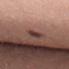Impression: Captured during whole-body skin photography for melanoma surveillance; the lesion was not biopsied. Context: Approximately 3 mm at its widest. Cropped from a whole-body photographic skin survey; the tile spans about 15 mm. This is a white-light tile. Automated tile analysis of the lesion measured two-axis asymmetry of about 0.2. The software also gave a border-irregularity index near 3/10 and a within-lesion color-variation index near 4/10. And it measured a classifier nevus-likeness of about 90/100. From the lower back. A female subject, about 30 years old.